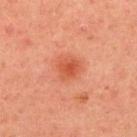Q: What kind of image is this?
A: ~15 mm crop, total-body skin-cancer survey
Q: Patient demographics?
A: male, roughly 45 years of age
Q: Automated lesion metrics?
A: an area of roughly 6 mm² and a shape eccentricity near 0.5; a within-lesion color-variation index near 2.5/10 and peripheral color asymmetry of about 1; a classifier nevus-likeness of about 60/100 and lesion-presence confidence of about 100/100
Q: What lighting was used for the tile?
A: cross-polarized illumination
Q: What is the anatomic site?
A: the upper back
Q: How large is the lesion?
A: ≈3 mm